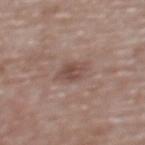Case summary:
• biopsy status — catalogued during a skin exam; not biopsied
• acquisition — 15 mm crop, total-body photography
• lesion size — ≈3 mm
• tile lighting — white-light
• subject — female, about 65 years old
• site — the back